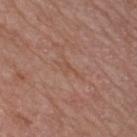Clinical impression:
Part of a total-body skin-imaging series; this lesion was reviewed on a skin check and was not flagged for biopsy.
Image and clinical context:
A close-up tile cropped from a whole-body skin photograph, about 15 mm across. The patient is a male approximately 50 years of age. Measured at roughly 3 mm in maximum diameter. From the chest. This is a white-light tile.